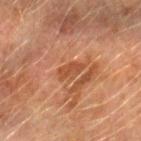Q: Was a biopsy performed?
A: total-body-photography surveillance lesion; no biopsy
Q: What lighting was used for the tile?
A: cross-polarized illumination
Q: Who is the patient?
A: male, aged approximately 65
Q: Lesion location?
A: the left forearm
Q: How was this image acquired?
A: ~15 mm crop, total-body skin-cancer survey
Q: What is the lesion's diameter?
A: ~3.5 mm (longest diameter)